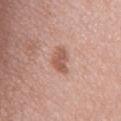follow-up — total-body-photography surveillance lesion; no biopsy
patient — female, aged 68–72
body site — the mid back
tile lighting — white-light
diameter — ≈3.5 mm
acquisition — ~15 mm tile from a whole-body skin photo